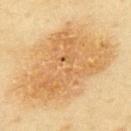workup: imaged on a skin check; not biopsied
patient: female, aged 58–62
lesion size: about 11.5 mm
tile lighting: cross-polarized illumination
imaging modality: 15 mm crop, total-body photography
automated lesion analysis: a nevus-likeness score of about 95/100 and a lesion-detection confidence of about 100/100
anatomic site: the back